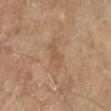Imaged during a routine full-body skin examination; the lesion was not biopsied and no histopathology is available. Cropped from a total-body skin-imaging series; the visible field is about 15 mm. From the left lower leg. The lesion-visualizer software estimated a footprint of about 5 mm² and two-axis asymmetry of about 0.45. The analysis additionally found a lesion color around L≈54 a*≈18 b*≈33 in CIELAB, roughly 6 lightness units darker than nearby skin, and a normalized lesion–skin contrast near 5. It also reported lesion-presence confidence of about 100/100. Imaged with cross-polarized lighting. The subject is a male aged around 65.